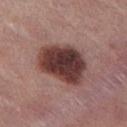  biopsy_status: not biopsied; imaged during a skin examination
  lighting: white-light
  site: leg
  image:
    source: total-body photography crop
    field_of_view_mm: 15
  patient:
    sex: female
    age_approx: 55